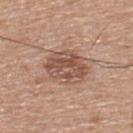follow-up: imaged on a skin check; not biopsied
patient: male, aged 53–57
location: the back
tile lighting: white-light
lesion size: about 5 mm
image: ~15 mm tile from a whole-body skin photo